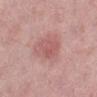Q: Is there a histopathology result?
A: imaged on a skin check; not biopsied
Q: How was this image acquired?
A: 15 mm crop, total-body photography
Q: How was the tile lit?
A: white-light
Q: Automated lesion metrics?
A: a mean CIELAB color near L≈57 a*≈26 b*≈23, roughly 8 lightness units darker than nearby skin, and a normalized border contrast of about 5.5; a classifier nevus-likeness of about 35/100 and a detector confidence of about 100 out of 100 that the crop contains a lesion
Q: What is the anatomic site?
A: the left lower leg
Q: Patient demographics?
A: female, in their 40s